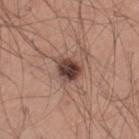Image and clinical context: A male subject aged around 40. The lesion-visualizer software estimated a mean CIELAB color near L≈41 a*≈19 b*≈23. The software also gave border irregularity of about 2.5 on a 0–10 scale, a color-variation rating of about 6/10, and peripheral color asymmetry of about 2. The analysis additionally found a nevus-likeness score of about 45/100. About 3 mm across. The tile uses white-light illumination. Located on the left thigh. A 15 mm close-up tile from a total-body photography series done for melanoma screening.